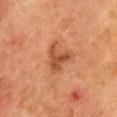No biopsy was performed on this lesion — it was imaged during a full skin examination and was not determined to be concerning. The lesion is located on the chest. The lesion-visualizer software estimated an area of roughly 7.5 mm², an outline eccentricity of about 0.4 (0 = round, 1 = elongated), and a symmetry-axis asymmetry near 0.6. The analysis additionally found a lesion color around L≈41 a*≈23 b*≈32 in CIELAB, about 9 CIELAB-L* units darker than the surrounding skin, and a normalized border contrast of about 7.5. The subject is a female roughly 50 years of age. A region of skin cropped from a whole-body photographic capture, roughly 15 mm wide. Approximately 3.5 mm at its widest.